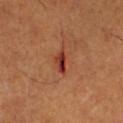The lesion was tiled from a total-body skin photograph and was not biopsied. Approximately 3 mm at its widest. On the left lower leg. A close-up tile cropped from a whole-body skin photograph, about 15 mm across. A male patient, aged 48–52.